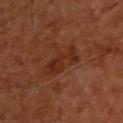Findings:
• follow-up · no biopsy performed (imaged during a skin exam)
• patient · male, in their mid-50s
• diameter · ≈4.5 mm
• site · the upper back
• tile lighting · cross-polarized
• image source · total-body-photography crop, ~15 mm field of view